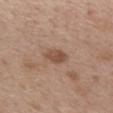Assessment:
This lesion was catalogued during total-body skin photography and was not selected for biopsy.
Context:
Cropped from a whole-body photographic skin survey; the tile spans about 15 mm. From the back. A female subject aged approximately 40.